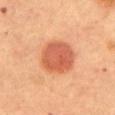No biopsy was performed on this lesion — it was imaged during a full skin examination and was not determined to be concerning. On the abdomen. The lesion's longest dimension is about 4.5 mm. A female patient, approximately 60 years of age. This image is a 15 mm lesion crop taken from a total-body photograph.